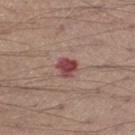Q: What lighting was used for the tile?
A: white-light
Q: Patient demographics?
A: male, in their 40s
Q: What is the lesion's diameter?
A: ~2.5 mm (longest diameter)
Q: Where on the body is the lesion?
A: the leg
Q: How was this image acquired?
A: ~15 mm tile from a whole-body skin photo